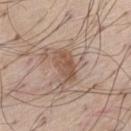Q: Was this lesion biopsied?
A: total-body-photography surveillance lesion; no biopsy
Q: What is the imaging modality?
A: ~15 mm crop, total-body skin-cancer survey
Q: Where on the body is the lesion?
A: the left thigh
Q: What are the patient's age and sex?
A: male, aged 68 to 72